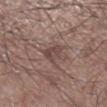biopsy status: no biopsy performed (imaged during a skin exam)
patient: male, in their 60s
illumination: white-light illumination
body site: the right lower leg
lesion size: ≈2.5 mm
automated lesion analysis: a mean CIELAB color near L≈44 a*≈17 b*≈19, roughly 8 lightness units darker than nearby skin, and a normalized border contrast of about 6.5; border irregularity of about 2.5 on a 0–10 scale, internal color variation of about 2.5 on a 0–10 scale, and peripheral color asymmetry of about 1
image: ~15 mm tile from a whole-body skin photo